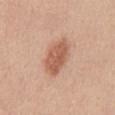biopsy_status: not biopsied; imaged during a skin examination
lighting: white-light
site: mid back
patient:
  sex: female
  age_approx: 45
automated_metrics:
  eccentricity: 0.9
  border_irregularity_0_10: 3.0
  color_variation_0_10: 3.0
  peripheral_color_asymmetry: 1.0
  nevus_likeness_0_100: 90
  lesion_detection_confidence_0_100: 100
image:
  source: total-body photography crop
  field_of_view_mm: 15